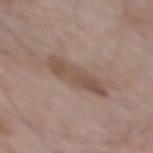notes — catalogued during a skin exam; not biopsied
lesion diameter — ≈6.5 mm
subject — male, approximately 65 years of age
imaging modality — total-body-photography crop, ~15 mm field of view
illumination — white-light illumination
body site — the upper back